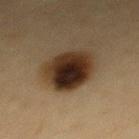{"lighting": "cross-polarized", "lesion_size": {"long_diameter_mm_approx": 5.0}, "image": {"source": "total-body photography crop", "field_of_view_mm": 15}, "site": "upper back", "patient": {"sex": "female", "age_approx": 40}}